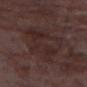  biopsy_status: not biopsied; imaged during a skin examination
  image:
    source: total-body photography crop
    field_of_view_mm: 15
  site: right arm
  lighting: white-light
  patient:
    sex: male
    age_approx: 80